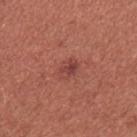The lesion was tiled from a total-body skin photograph and was not biopsied.
Located on the right upper arm.
An algorithmic analysis of the crop reported an area of roughly 4 mm². The analysis additionally found a within-lesion color-variation index near 4/10.
The recorded lesion diameter is about 2.5 mm.
A 15 mm close-up tile from a total-body photography series done for melanoma screening.
A female subject, aged around 35.